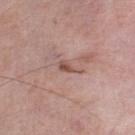Q: Is there a histopathology result?
A: imaged on a skin check; not biopsied